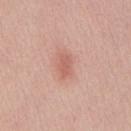Impression:
The lesion was photographed on a routine skin check and not biopsied; there is no pathology result.
Background:
Automated tile analysis of the lesion measured an average lesion color of about L≈60 a*≈26 b*≈27 (CIELAB) and about 9 CIELAB-L* units darker than the surrounding skin. The software also gave a border-irregularity index near 2.5/10, internal color variation of about 1 on a 0–10 scale, and a peripheral color-asymmetry measure near 0.5. It also reported an automated nevus-likeness rating near 35 out of 100 and a lesion-detection confidence of about 100/100. This is a white-light tile. On the front of the torso. A close-up tile cropped from a whole-body skin photograph, about 15 mm across. A male patient, aged 38–42.